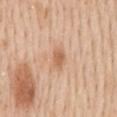– follow-up · imaged on a skin check; not biopsied
– lesion size · ≈2.5 mm
– anatomic site · the mid back
– imaging modality · ~15 mm tile from a whole-body skin photo
– patient · male, aged 58–62
– automated metrics · an eccentricity of roughly 0.8 and a symmetry-axis asymmetry near 0.25; an average lesion color of about L≈62 a*≈22 b*≈35 (CIELAB) and a normalized border contrast of about 6.5; border irregularity of about 2 on a 0–10 scale, a within-lesion color-variation index near 1.5/10, and peripheral color asymmetry of about 0.5